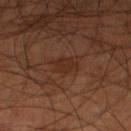The lesion was photographed on a routine skin check and not biopsied; there is no pathology result. From the left lower leg. Cropped from a total-body skin-imaging series; the visible field is about 15 mm. Captured under cross-polarized illumination. The total-body-photography lesion software estimated a border-irregularity rating of about 3/10, internal color variation of about 2 on a 0–10 scale, and a peripheral color-asymmetry measure near 0.5. A male subject aged 53–57.